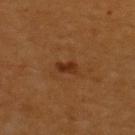workup = no biopsy performed (imaged during a skin exam); acquisition = 15 mm crop, total-body photography; anatomic site = the upper back; subject = male, roughly 60 years of age.